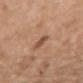<record>
  <biopsy_status>not biopsied; imaged during a skin examination</biopsy_status>
  <lesion_size>
    <long_diameter_mm_approx>2.5</long_diameter_mm_approx>
  </lesion_size>
  <lighting>white-light</lighting>
  <patient>
    <sex>female</sex>
    <age_approx>55</age_approx>
  </patient>
  <automated_metrics>
    <area_mm2_approx>3.5</area_mm2_approx>
    <eccentricity>0.85</eccentricity>
    <lesion_detection_confidence_0_100>100</lesion_detection_confidence_0_100>
  </automated_metrics>
  <image>
    <source>total-body photography crop</source>
    <field_of_view_mm>15</field_of_view_mm>
  </image>
  <site>upper back</site>
</record>